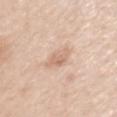biopsy status: total-body-photography surveillance lesion; no biopsy
acquisition: ~15 mm crop, total-body skin-cancer survey
anatomic site: the left upper arm
image-analysis metrics: a footprint of about 5 mm², an eccentricity of roughly 0.7, and a shape-asymmetry score of about 0.3 (0 = symmetric); an automated nevus-likeness rating near 0 out of 100 and a detector confidence of about 100 out of 100 that the crop contains a lesion
lesion size: ≈3 mm
subject: male, approximately 60 years of age